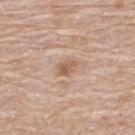workup=imaged on a skin check; not biopsied | imaging modality=~15 mm crop, total-body skin-cancer survey | subject=male, aged approximately 80 | body site=the upper back.